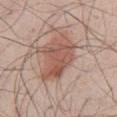  biopsy_status: not biopsied; imaged during a skin examination
  image:
    source: total-body photography crop
    field_of_view_mm: 15
  site: abdomen
  patient:
    sex: male
    age_approx: 50